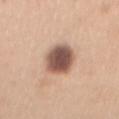Part of a total-body skin-imaging series; this lesion was reviewed on a skin check and was not flagged for biopsy. A female patient, about 30 years old. The recorded lesion diameter is about 5.5 mm. The tile uses white-light illumination. This image is a 15 mm lesion crop taken from a total-body photograph. The lesion is located on the chest. The total-body-photography lesion software estimated a lesion area of about 13 mm², an outline eccentricity of about 0.85 (0 = round, 1 = elongated), and a shape-asymmetry score of about 0.15 (0 = symmetric). The software also gave a mean CIELAB color near L≈54 a*≈19 b*≈26, roughly 19 lightness units darker than nearby skin, and a normalized border contrast of about 12. The analysis additionally found a border-irregularity index near 2/10 and radial color variation of about 1.5.